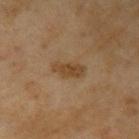Captured during whole-body skin photography for melanoma surveillance; the lesion was not biopsied. The patient is a female roughly 60 years of age. A 15 mm crop from a total-body photograph taken for skin-cancer surveillance. From the left upper arm. Automated image analysis of the tile measured a lesion color around L≈40 a*≈15 b*≈32 in CIELAB, about 7 CIELAB-L* units darker than the surrounding skin, and a lesion-to-skin contrast of about 7 (normalized; higher = more distinct). The software also gave a border-irregularity rating of about 3.5/10 and a within-lesion color-variation index near 2/10. The software also gave a nevus-likeness score of about 5/100 and lesion-presence confidence of about 100/100. The tile uses cross-polarized illumination. Approximately 4 mm at its widest.